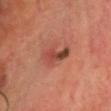Clinical impression:
The lesion was tiled from a total-body skin photograph and was not biopsied.
Context:
Imaged with cross-polarized lighting. Cropped from a whole-body photographic skin survey; the tile spans about 15 mm. A male subject, roughly 70 years of age. The lesion is located on the head or neck. Approximately 5 mm at its widest.